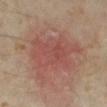follow-up: total-body-photography surveillance lesion; no biopsy
image: total-body-photography crop, ~15 mm field of view
body site: the right lower leg
image-analysis metrics: an area of roughly 26 mm² and an outline eccentricity of about 0.8 (0 = round, 1 = elongated); a border-irregularity index near 4.5/10, a within-lesion color-variation index near 3/10, and a peripheral color-asymmetry measure near 1; an automated nevus-likeness rating near 0 out of 100 and lesion-presence confidence of about 100/100
diameter: about 8 mm
subject: female, about 35 years old